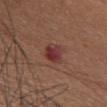follow-up: no biopsy performed (imaged during a skin exam)
subject: female, about 65 years old
anatomic site: the chest
acquisition: ~15 mm tile from a whole-body skin photo
illumination: white-light
size: ~3 mm (longest diameter)